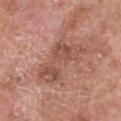Case summary:
* TBP lesion metrics — a lesion color around L≈51 a*≈23 b*≈27 in CIELAB and a normalized lesion–skin contrast near 6.5
* image — total-body-photography crop, ~15 mm field of view
* site — the chest
* illumination — white-light illumination
* lesion diameter — ~6.5 mm (longest diameter)
* subject — female, aged 73–77